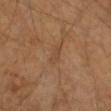workup: total-body-photography surveillance lesion; no biopsy | patient: aged 53 to 57 | site: the right forearm | automated lesion analysis: a lesion area of about 3 mm² and an outline eccentricity of about 0.9 (0 = round, 1 = elongated) | acquisition: total-body-photography crop, ~15 mm field of view | lesion diameter: about 2.5 mm.